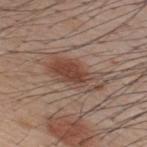* subject — male, approximately 35 years of age
* body site — the upper back
* acquisition — 15 mm crop, total-body photography